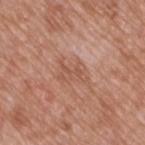Recorded during total-body skin imaging; not selected for excision or biopsy.
Longest diameter approximately 4 mm.
The subject is a male aged 48 to 52.
The lesion is located on the upper back.
This image is a 15 mm lesion crop taken from a total-body photograph.